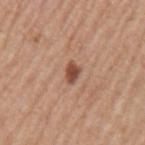biopsy status: catalogued during a skin exam; not biopsied | anatomic site: the left upper arm | patient: male, roughly 65 years of age | acquisition: total-body-photography crop, ~15 mm field of view.